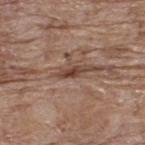Notes:
• biopsy status: catalogued during a skin exam; not biopsied
• image: ~15 mm crop, total-body skin-cancer survey
• lighting: white-light
• automated lesion analysis: a lesion area of about 3 mm² and an outline eccentricity of about 0.9 (0 = round, 1 = elongated); a nevus-likeness score of about 5/100 and a lesion-detection confidence of about 85/100
• anatomic site: the upper back
• subject: male, about 70 years old
• diameter: ≈3 mm